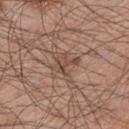Impression: This lesion was catalogued during total-body skin photography and was not selected for biopsy. Clinical summary: The lesion-visualizer software estimated an area of roughly 5 mm², an outline eccentricity of about 0.65 (0 = round, 1 = elongated), and two-axis asymmetry of about 0.55. And it measured an average lesion color of about L≈47 a*≈19 b*≈26 (CIELAB) and a lesion-to-skin contrast of about 6.5 (normalized; higher = more distinct). A male subject, about 45 years old. Imaged with white-light lighting. From the left upper arm. A lesion tile, about 15 mm wide, cut from a 3D total-body photograph. The lesion's longest dimension is about 3 mm.